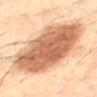biopsy_status: not biopsied; imaged during a skin examination
automated_metrics:
  border_irregularity_0_10: 2.0
  color_variation_0_10: 5.0
  nevus_likeness_0_100: 90
  lesion_detection_confidence_0_100: 100
patient:
  sex: male
  age_approx: 50
lighting: cross-polarized
site: mid back
lesion_size:
  long_diameter_mm_approx: 10.5
image:
  source: total-body photography crop
  field_of_view_mm: 15